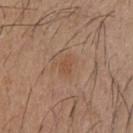Q: Is there a histopathology result?
A: catalogued during a skin exam; not biopsied
Q: What kind of image is this?
A: total-body-photography crop, ~15 mm field of view
Q: Patient demographics?
A: male, aged 28 to 32
Q: Illumination type?
A: white-light illumination
Q: Automated lesion metrics?
A: a lesion area of about 3 mm² and two-axis asymmetry of about 0.25
Q: What is the anatomic site?
A: the upper back